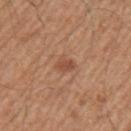Clinical impression:
This lesion was catalogued during total-body skin photography and was not selected for biopsy.
Acquisition and patient details:
A roughly 15 mm field-of-view crop from a total-body skin photograph. Approximately 2.5 mm at its widest. From the arm. A male patient approximately 65 years of age.